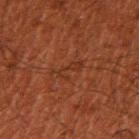workup: no biopsy performed (imaged during a skin exam)
acquisition: 15 mm crop, total-body photography
site: the right upper arm
image-analysis metrics: a lesion-detection confidence of about 95/100
subject: male, in their 50s